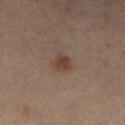Case summary:
• notes · no biopsy performed (imaged during a skin exam)
• acquisition · ~15 mm crop, total-body skin-cancer survey
• size · ~2.5 mm (longest diameter)
• tile lighting · cross-polarized illumination
• subject · male, roughly 55 years of age
• anatomic site · the right forearm